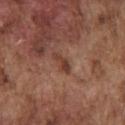Q: Was a biopsy performed?
A: catalogued during a skin exam; not biopsied
Q: What kind of image is this?
A: ~15 mm tile from a whole-body skin photo
Q: What is the anatomic site?
A: the front of the torso
Q: Lesion size?
A: about 3 mm
Q: Patient demographics?
A: male, about 75 years old